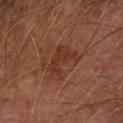Assessment: Captured during whole-body skin photography for melanoma surveillance; the lesion was not biopsied. Acquisition and patient details: Measured at roughly 4.5 mm in maximum diameter. The tile uses cross-polarized illumination. From the left forearm. A close-up tile cropped from a whole-body skin photograph, about 15 mm across. A male patient, aged 73 to 77.